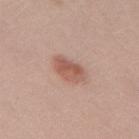  patient:
    sex: male
    age_approx: 30
  lesion_size:
    long_diameter_mm_approx: 4.0
  lighting: white-light
  automated_metrics:
    vs_skin_darker_L: 11.0
    vs_skin_contrast_norm: 7.5
  image:
    source: total-body photography crop
    field_of_view_mm: 15